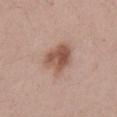Part of a total-body skin-imaging series; this lesion was reviewed on a skin check and was not flagged for biopsy. The lesion is located on the abdomen. An algorithmic analysis of the crop reported an area of roughly 10 mm² and an outline eccentricity of about 0.55 (0 = round, 1 = elongated). And it measured a mean CIELAB color near L≈53 a*≈21 b*≈27 and roughly 12 lightness units darker than nearby skin. The patient is a male aged 38–42. This is a white-light tile. Measured at roughly 4 mm in maximum diameter. A close-up tile cropped from a whole-body skin photograph, about 15 mm across.